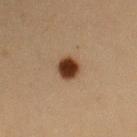Image and clinical context:
Imaged with cross-polarized lighting. Cropped from a whole-body photographic skin survey; the tile spans about 15 mm. The lesion is located on the right upper arm. The subject is a female roughly 40 years of age. Approximately 3 mm at its widest.